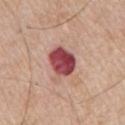Clinical impression: No biopsy was performed on this lesion — it was imaged during a full skin examination and was not determined to be concerning. Background: Cropped from a whole-body photographic skin survey; the tile spans about 15 mm. Captured under white-light illumination. A male subject, in their mid-60s. The lesion is on the right upper arm. Longest diameter approximately 4.5 mm.